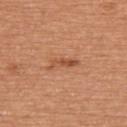follow-up = no biopsy performed (imaged during a skin exam) | size = about 4 mm | subject = female, approximately 75 years of age | image = 15 mm crop, total-body photography | automated lesion analysis = a lesion area of about 4 mm² and a shape eccentricity near 0.95 | illumination = white-light illumination | location = the upper back.